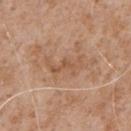workup=no biopsy performed (imaged during a skin exam) | TBP lesion metrics=a lesion color around L≈55 a*≈20 b*≈33 in CIELAB, about 7 CIELAB-L* units darker than the surrounding skin, and a normalized lesion–skin contrast near 5.5; peripheral color asymmetry of about 0.5; a detector confidence of about 95 out of 100 that the crop contains a lesion | patient=male, aged around 65 | lighting=white-light illumination | location=the chest | diameter=about 4.5 mm | acquisition=~15 mm crop, total-body skin-cancer survey.